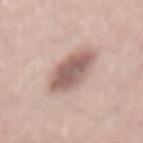This lesion was catalogued during total-body skin photography and was not selected for biopsy. A male patient, in their 60s. About 5.5 mm across. This is a white-light tile. Automated tile analysis of the lesion measured an average lesion color of about L≈58 a*≈18 b*≈23 (CIELAB), roughly 14 lightness units darker than nearby skin, and a normalized border contrast of about 9. Cropped from a whole-body photographic skin survey; the tile spans about 15 mm. Located on the mid back.